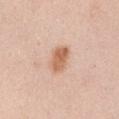Q: Was a biopsy performed?
A: imaged on a skin check; not biopsied
Q: Where on the body is the lesion?
A: the abdomen
Q: How was the tile lit?
A: white-light
Q: How large is the lesion?
A: ≈3.5 mm
Q: What are the patient's age and sex?
A: female, aged approximately 50
Q: What is the imaging modality?
A: ~15 mm tile from a whole-body skin photo